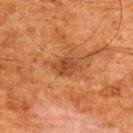Part of a total-body skin-imaging series; this lesion was reviewed on a skin check and was not flagged for biopsy. On the upper back. The lesion's longest dimension is about 3 mm. The subject is a male approximately 65 years of age. A 15 mm crop from a total-body photograph taken for skin-cancer surveillance.